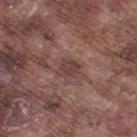Impression: No biopsy was performed on this lesion — it was imaged during a full skin examination and was not determined to be concerning. Clinical summary: A region of skin cropped from a whole-body photographic capture, roughly 15 mm wide. A male subject aged 73 to 77. From the left thigh.